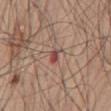The lesion was tiled from a total-body skin photograph and was not biopsied.
Located on the mid back.
This image is a 15 mm lesion crop taken from a total-body photograph.
The patient is a male roughly 70 years of age.